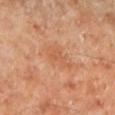Findings:
• workup · catalogued during a skin exam; not biopsied
• patient · male, aged around 70
• site · the left lower leg
• size · ≈3.5 mm
• image · 15 mm crop, total-body photography